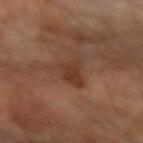Clinical summary:
A male subject roughly 70 years of age. Captured under cross-polarized illumination. Automated tile analysis of the lesion measured a lesion color around L≈36 a*≈19 b*≈28 in CIELAB. The software also gave a border-irregularity index near 5.5/10. The analysis additionally found a nevus-likeness score of about 20/100 and a lesion-detection confidence of about 100/100. Located on the left forearm. A 15 mm close-up extracted from a 3D total-body photography capture.